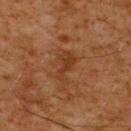The lesion was tiled from a total-body skin photograph and was not biopsied. From the upper back. A male patient aged around 60. Imaged with cross-polarized lighting. Measured at roughly 3.5 mm in maximum diameter. A close-up tile cropped from a whole-body skin photograph, about 15 mm across. Automated tile analysis of the lesion measured an area of roughly 5 mm², a shape eccentricity near 0.9, and a shape-asymmetry score of about 0.45 (0 = symmetric). The software also gave about 6 CIELAB-L* units darker than the surrounding skin and a normalized border contrast of about 6.5. The software also gave an automated nevus-likeness rating near 0 out of 100 and lesion-presence confidence of about 100/100.